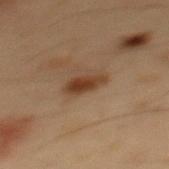Q: What did automated image analysis measure?
A: two-axis asymmetry of about 0.25; a classifier nevus-likeness of about 95/100 and lesion-presence confidence of about 100/100
Q: How was this image acquired?
A: total-body-photography crop, ~15 mm field of view
Q: Where on the body is the lesion?
A: the mid back
Q: What lighting was used for the tile?
A: cross-polarized illumination
Q: Patient demographics?
A: male, aged 53–57
Q: Lesion size?
A: ≈3 mm